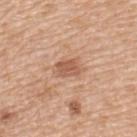Case summary:
• biopsy status · total-body-photography surveillance lesion; no biopsy
• acquisition · 15 mm crop, total-body photography
• lesion size · about 2.5 mm
• automated metrics · an average lesion color of about L≈57 a*≈22 b*≈32 (CIELAB); a detector confidence of about 100 out of 100 that the crop contains a lesion
• illumination · white-light
• subject · female, aged approximately 55
• body site · the back